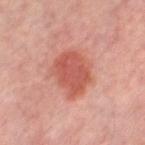Imaged during a routine full-body skin examination; the lesion was not biopsied and no histopathology is available. A close-up tile cropped from a whole-body skin photograph, about 15 mm across. Longest diameter approximately 5 mm. Automated tile analysis of the lesion measured an eccentricity of roughly 0.7 and a symmetry-axis asymmetry near 0.2. The software also gave an average lesion color of about L≈53 a*≈30 b*≈29 (CIELAB), roughly 11 lightness units darker than nearby skin, and a lesion-to-skin contrast of about 7.5 (normalized; higher = more distinct). The software also gave an automated nevus-likeness rating near 100 out of 100 and lesion-presence confidence of about 100/100. From the left thigh. A female patient in their 50s.